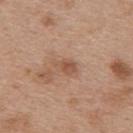| field | value |
|---|---|
| notes | catalogued during a skin exam; not biopsied |
| site | the upper back |
| tile lighting | white-light |
| automated lesion analysis | a mean CIELAB color near L≈53 a*≈21 b*≈30, a lesion–skin lightness drop of about 9, and a normalized lesion–skin contrast near 6; an automated nevus-likeness rating near 0 out of 100 |
| image | ~15 mm crop, total-body skin-cancer survey |
| lesion size | about 3 mm |
| subject | female, approximately 40 years of age |